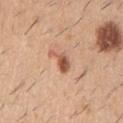Impression:
The lesion was tiled from a total-body skin photograph and was not biopsied.
Background:
The patient is a male in their 60s. Captured under white-light illumination. A roughly 15 mm field-of-view crop from a total-body skin photograph. Automated tile analysis of the lesion measured a lesion color around L≈56 a*≈24 b*≈32 in CIELAB, about 14 CIELAB-L* units darker than the surrounding skin, and a normalized border contrast of about 8.5. It also reported a border-irregularity index near 5.5/10 and internal color variation of about 3.5 on a 0–10 scale. About 3 mm across. The lesion is located on the left upper arm.